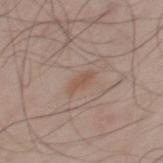Recorded during total-body skin imaging; not selected for excision or biopsy.
The total-body-photography lesion software estimated a shape eccentricity near 0.9 and two-axis asymmetry of about 0.25. And it measured a border-irregularity rating of about 3/10, a within-lesion color-variation index near 0/10, and radial color variation of about 0.
Imaged with white-light lighting.
This image is a 15 mm lesion crop taken from a total-body photograph.
Approximately 2.5 mm at its widest.
On the upper back.
A male subject, about 45 years old.